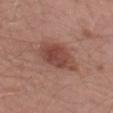biopsy status: total-body-photography surveillance lesion; no biopsy | imaging modality: ~15 mm tile from a whole-body skin photo | size: about 6 mm | subject: male, approximately 65 years of age | anatomic site: the left thigh.